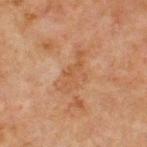No biopsy was performed on this lesion — it was imaged during a full skin examination and was not determined to be concerning. A 15 mm close-up extracted from a 3D total-body photography capture. This is a cross-polarized tile. Automated image analysis of the tile measured an average lesion color of about L≈43 a*≈18 b*≈30 (CIELAB), a lesion–skin lightness drop of about 5, and a lesion-to-skin contrast of about 5 (normalized; higher = more distinct). The analysis additionally found border irregularity of about 6 on a 0–10 scale. The patient is a male in their mid-60s. On the chest. Approximately 5 mm at its widest.